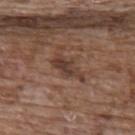Q: Was a biopsy performed?
A: total-body-photography surveillance lesion; no biopsy
Q: What is the imaging modality?
A: ~15 mm crop, total-body skin-cancer survey
Q: What are the patient's age and sex?
A: female, in their mid- to late 60s
Q: Lesion location?
A: the upper back